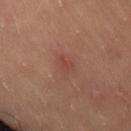Captured during whole-body skin photography for melanoma surveillance; the lesion was not biopsied.
About 2.5 mm across.
A 15 mm crop from a total-body photograph taken for skin-cancer surveillance.
From the back.
The patient is a female in their mid- to late 30s.
The tile uses cross-polarized illumination.